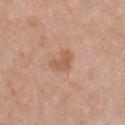This lesion was catalogued during total-body skin photography and was not selected for biopsy.
Captured under white-light illumination.
A 15 mm close-up extracted from a 3D total-body photography capture.
Approximately 3 mm at its widest.
A female patient, aged 38 to 42.
From the chest.